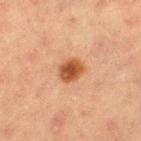Clinical impression: The lesion was tiled from a total-body skin photograph and was not biopsied.